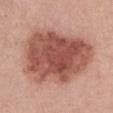A female patient, roughly 35 years of age. Measured at roughly 9.5 mm in maximum diameter. On the front of the torso. The lesion-visualizer software estimated an area of roughly 55 mm², an outline eccentricity of about 0.7 (0 = round, 1 = elongated), and a symmetry-axis asymmetry near 0.25. The software also gave an average lesion color of about L≈52 a*≈26 b*≈27 (CIELAB) and about 15 CIELAB-L* units darker than the surrounding skin. This is a white-light tile. This image is a 15 mm lesion crop taken from a total-body photograph.